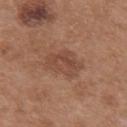Imaged with white-light lighting.
Located on the upper back.
A female patient, aged approximately 40.
Automated image analysis of the tile measured an eccentricity of roughly 0.75 and two-axis asymmetry of about 0.3. And it measured a nevus-likeness score of about 0/100 and lesion-presence confidence of about 100/100.
Cropped from a total-body skin-imaging series; the visible field is about 15 mm.